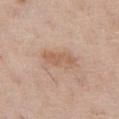Q: Was a biopsy performed?
A: catalogued during a skin exam; not biopsied
Q: What is the anatomic site?
A: the left thigh
Q: How was the tile lit?
A: white-light
Q: Lesion size?
A: ~4.5 mm (longest diameter)
Q: What kind of image is this?
A: 15 mm crop, total-body photography
Q: Patient demographics?
A: female, approximately 60 years of age
Q: Automated lesion metrics?
A: a shape eccentricity near 0.9 and a symmetry-axis asymmetry near 0.2; roughly 8 lightness units darker than nearby skin and a normalized lesion–skin contrast near 6; an automated nevus-likeness rating near 10 out of 100 and lesion-presence confidence of about 100/100